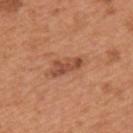Q: Was a biopsy performed?
A: catalogued during a skin exam; not biopsied
Q: Automated lesion metrics?
A: a lesion area of about 5.5 mm², an eccentricity of roughly 0.95, and a shape-asymmetry score of about 0.35 (0 = symmetric); a mean CIELAB color near L≈49 a*≈26 b*≈33, a lesion–skin lightness drop of about 11, and a normalized lesion–skin contrast near 7.5
Q: Who is the patient?
A: male, roughly 65 years of age
Q: How was the tile lit?
A: white-light illumination
Q: Lesion size?
A: ~4.5 mm (longest diameter)
Q: How was this image acquired?
A: 15 mm crop, total-body photography
Q: What is the anatomic site?
A: the arm